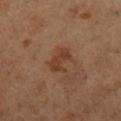biopsy status — catalogued during a skin exam; not biopsied | site — the left lower leg | patient — female, about 55 years old | automated metrics — a lesion area of about 6 mm², an outline eccentricity of about 0.75 (0 = round, 1 = elongated), and two-axis asymmetry of about 0.3; a classifier nevus-likeness of about 35/100 and a lesion-detection confidence of about 100/100 | size — ~3.5 mm (longest diameter) | acquisition — ~15 mm crop, total-body skin-cancer survey.